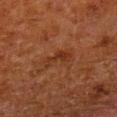Part of a total-body skin-imaging series; this lesion was reviewed on a skin check and was not flagged for biopsy. The subject is a male in their 80s. A close-up tile cropped from a whole-body skin photograph, about 15 mm across. The lesion is on the left lower leg. The tile uses cross-polarized illumination.